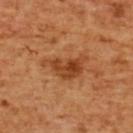Impression:
Part of a total-body skin-imaging series; this lesion was reviewed on a skin check and was not flagged for biopsy.
Clinical summary:
A female subject aged around 55. Cropped from a whole-body photographic skin survey; the tile spans about 15 mm. On the upper back. The lesion's longest dimension is about 5.5 mm. This is a cross-polarized tile.